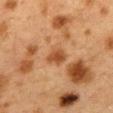follow-up: total-body-photography surveillance lesion; no biopsy | image: total-body-photography crop, ~15 mm field of view | patient: female, aged 38–42 | location: the mid back.